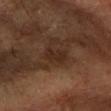| key | value |
|---|---|
| biopsy status | total-body-photography surveillance lesion; no biopsy |
| lighting | cross-polarized illumination |
| TBP lesion metrics | a lesion area of about 4 mm², an eccentricity of roughly 0.75, and a symmetry-axis asymmetry near 0.25; a within-lesion color-variation index near 2/10 |
| patient | male, aged around 60 |
| body site | the left forearm |
| image | ~15 mm crop, total-body skin-cancer survey |